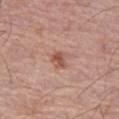A lesion tile, about 15 mm wide, cut from a 3D total-body photograph. From the left thigh. An algorithmic analysis of the crop reported a lesion area of about 4 mm², an outline eccentricity of about 0.55 (0 = round, 1 = elongated), and a symmetry-axis asymmetry near 0.35. The analysis additionally found border irregularity of about 3 on a 0–10 scale, a within-lesion color-variation index near 3/10, and peripheral color asymmetry of about 1. A male subject about 75 years old.